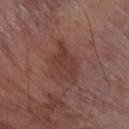| feature | finding |
|---|---|
| biopsy status | catalogued during a skin exam; not biopsied |
| site | the arm |
| image | 15 mm crop, total-body photography |
| subject | male, aged around 75 |
| image-analysis metrics | a footprint of about 12 mm², a shape eccentricity near 0.75, and a shape-asymmetry score of about 0.35 (0 = symmetric); a border-irregularity rating of about 4.5/10; a classifier nevus-likeness of about 0/100 and a lesion-detection confidence of about 100/100 |
| size | ≈5.5 mm |
| lighting | white-light |